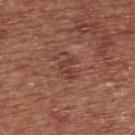Part of a total-body skin-imaging series; this lesion was reviewed on a skin check and was not flagged for biopsy. A lesion tile, about 15 mm wide, cut from a 3D total-body photograph. The subject is a male roughly 65 years of age. The lesion is located on the upper back.